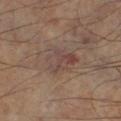Notes:
• workup · catalogued during a skin exam; not biopsied
• tile lighting · cross-polarized
• subject · male, about 60 years old
• lesion size · ~4 mm (longest diameter)
• image · total-body-photography crop, ~15 mm field of view
• site · the left lower leg